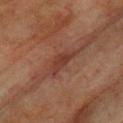Impression:
Recorded during total-body skin imaging; not selected for excision or biopsy.